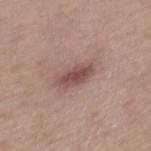{
  "biopsy_status": "not biopsied; imaged during a skin examination",
  "patient": {
    "sex": "female",
    "age_approx": 50
  },
  "site": "mid back",
  "automated_metrics": {
    "cielab_L": 50,
    "cielab_a": 19,
    "cielab_b": 22,
    "vs_skin_darker_L": 11.0,
    "vs_skin_contrast_norm": 8.0,
    "border_irregularity_0_10": 2.0,
    "color_variation_0_10": 3.0,
    "peripheral_color_asymmetry": 1.0,
    "nevus_likeness_0_100": 50,
    "lesion_detection_confidence_0_100": 100
  },
  "lesion_size": {
    "long_diameter_mm_approx": 4.0
  },
  "lighting": "white-light",
  "image": {
    "source": "total-body photography crop",
    "field_of_view_mm": 15
  }
}